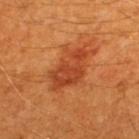Clinical impression:
Part of a total-body skin-imaging series; this lesion was reviewed on a skin check and was not flagged for biopsy.
Acquisition and patient details:
An algorithmic analysis of the crop reported a footprint of about 16 mm², a shape eccentricity near 0.85, and two-axis asymmetry of about 0.35. The analysis additionally found a mean CIELAB color near L≈42 a*≈30 b*≈39 and about 9 CIELAB-L* units darker than the surrounding skin. The analysis additionally found a border-irregularity rating of about 4.5/10, a within-lesion color-variation index near 4/10, and a peripheral color-asymmetry measure near 1.5. And it measured a classifier nevus-likeness of about 40/100 and a lesion-detection confidence of about 100/100. This is a cross-polarized tile. A close-up tile cropped from a whole-body skin photograph, about 15 mm across. Located on the back. A male subject, in their 60s. Longest diameter approximately 6.5 mm.